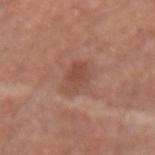Part of a total-body skin-imaging series; this lesion was reviewed on a skin check and was not flagged for biopsy.